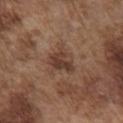The lesion was tiled from a total-body skin photograph and was not biopsied.
Located on the chest.
About 3.5 mm across.
A 15 mm close-up extracted from a 3D total-body photography capture.
This is a white-light tile.
A male patient in their mid- to late 70s.
The lesion-visualizer software estimated a footprint of about 8.5 mm², an eccentricity of roughly 0.4, and two-axis asymmetry of about 0.35. The software also gave a lesion color around L≈40 a*≈18 b*≈26 in CIELAB, roughly 9 lightness units darker than nearby skin, and a normalized border contrast of about 7.5. The software also gave border irregularity of about 3.5 on a 0–10 scale, a within-lesion color-variation index near 4/10, and a peripheral color-asymmetry measure near 1.5.